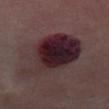The lesion was photographed on a routine skin check and not biopsied; there is no pathology result. The lesion is on the chest. A female patient, aged 53 to 57. The lesion's longest dimension is about 8.5 mm. This image is a 15 mm lesion crop taken from a total-body photograph. Automated tile analysis of the lesion measured a border-irregularity rating of about 3.5/10, a color-variation rating of about 9/10, and peripheral color asymmetry of about 2.5. The analysis additionally found an automated nevus-likeness rating near 0 out of 100 and lesion-presence confidence of about 100/100.